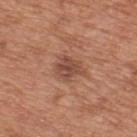<case>
  <biopsy_status>not biopsied; imaged during a skin examination</biopsy_status>
  <patient>
    <sex>male</sex>
    <age_approx>65</age_approx>
  </patient>
  <image>
    <source>total-body photography crop</source>
    <field_of_view_mm>15</field_of_view_mm>
  </image>
  <site>upper back</site>
  <automated_metrics>
    <area_mm2_approx>6.5</area_mm2_approx>
    <eccentricity>0.65</eccentricity>
    <shape_asymmetry>0.25</shape_asymmetry>
    <vs_skin_darker_L>10.0</vs_skin_darker_L>
    <vs_skin_contrast_norm>7.5</vs_skin_contrast_norm>
    <border_irregularity_0_10>3.0</border_irregularity_0_10>
    <color_variation_0_10>3.5</color_variation_0_10>
    <peripheral_color_asymmetry>1.5</peripheral_color_asymmetry>
    <nevus_likeness_0_100>15</nevus_likeness_0_100>
    <lesion_detection_confidence_0_100>100</lesion_detection_confidence_0_100>
  </automated_metrics>
  <lesion_size>
    <long_diameter_mm_approx>3.5</long_diameter_mm_approx>
  </lesion_size>
</case>